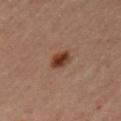• workup: catalogued during a skin exam; not biopsied